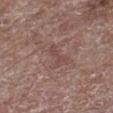The lesion was photographed on a routine skin check and not biopsied; there is no pathology result. The recorded lesion diameter is about 3 mm. This is a white-light tile. This image is a 15 mm lesion crop taken from a total-body photograph. A male patient, aged approximately 70. From the leg.